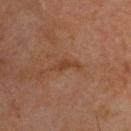No biopsy was performed on this lesion — it was imaged during a full skin examination and was not determined to be concerning. Cropped from a total-body skin-imaging series; the visible field is about 15 mm. The lesion is on the head or neck. Approximately 3.5 mm at its widest. The subject is a male about 70 years old. Automated tile analysis of the lesion measured a lesion color around L≈39 a*≈21 b*≈31 in CIELAB and a normalized lesion–skin contrast near 6.5. The analysis additionally found a nevus-likeness score of about 0/100 and a lesion-detection confidence of about 100/100. Captured under cross-polarized illumination.